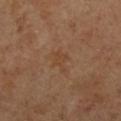biopsy_status: not biopsied; imaged during a skin examination
lighting: cross-polarized
lesion_size:
  long_diameter_mm_approx: 3.0
site: left lower leg
image:
  source: total-body photography crop
  field_of_view_mm: 15
automated_metrics:
  border_irregularity_0_10: 3.5
  color_variation_0_10: 1.0
patient:
  sex: female
  age_approx: 55